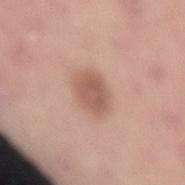<tbp_lesion>
<biopsy_status>not biopsied; imaged during a skin examination</biopsy_status>
</tbp_lesion>